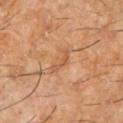Assessment:
This lesion was catalogued during total-body skin photography and was not selected for biopsy.
Image and clinical context:
Cropped from a total-body skin-imaging series; the visible field is about 15 mm. Measured at roughly 3.5 mm in maximum diameter. A male subject, in their 60s. Imaged with cross-polarized lighting. From the left lower leg. The lesion-visualizer software estimated a mean CIELAB color near L≈52 a*≈22 b*≈35 and roughly 7 lightness units darker than nearby skin. The software also gave a nevus-likeness score of about 0/100 and a detector confidence of about 90 out of 100 that the crop contains a lesion.